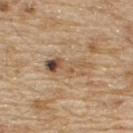follow-up=total-body-photography surveillance lesion; no biopsy
tile lighting=white-light illumination
diameter=≈5.5 mm
patient=male, aged around 70
imaging modality=~15 mm crop, total-body skin-cancer survey
automated metrics=a border-irregularity rating of about 5/10, a color-variation rating of about 9.5/10, and a peripheral color-asymmetry measure near 3
body site=the back